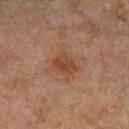The lesion was photographed on a routine skin check and not biopsied; there is no pathology result.
The subject is a male aged approximately 65.
A lesion tile, about 15 mm wide, cut from a 3D total-body photograph.
Imaged with cross-polarized lighting.
The lesion is on the left lower leg.
Automated image analysis of the tile measured a footprint of about 6 mm², a shape eccentricity near 0.5, and a shape-asymmetry score of about 0.25 (0 = symmetric). It also reported a lesion color around L≈34 a*≈19 b*≈26 in CIELAB and about 7 CIELAB-L* units darker than the surrounding skin. And it measured a nevus-likeness score of about 60/100 and a detector confidence of about 100 out of 100 that the crop contains a lesion.